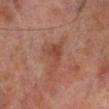Part of a total-body skin-imaging series; this lesion was reviewed on a skin check and was not flagged for biopsy.
Captured under cross-polarized illumination.
The lesion is on the right lower leg.
A male patient, roughly 70 years of age.
A 15 mm close-up extracted from a 3D total-body photography capture.
An algorithmic analysis of the crop reported border irregularity of about 5.5 on a 0–10 scale, a within-lesion color-variation index near 3.5/10, and peripheral color asymmetry of about 1.
Approximately 4 mm at its widest.